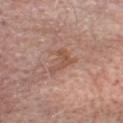Q: Was this lesion biopsied?
A: catalogued during a skin exam; not biopsied
Q: What is the anatomic site?
A: the head or neck
Q: Lesion size?
A: ~3.5 mm (longest diameter)
Q: What are the patient's age and sex?
A: female, aged 73 to 77
Q: How was the tile lit?
A: white-light
Q: What did automated image analysis measure?
A: an average lesion color of about L≈52 a*≈22 b*≈28 (CIELAB) and a normalized lesion–skin contrast near 6.5
Q: What kind of image is this?
A: ~15 mm crop, total-body skin-cancer survey